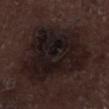{
  "biopsy_status": "not biopsied; imaged during a skin examination"
}